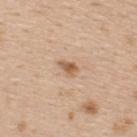Assessment: This lesion was catalogued during total-body skin photography and was not selected for biopsy.